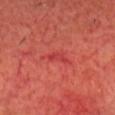On the head or neck. Imaged with cross-polarized lighting. A 15 mm close-up tile from a total-body photography series done for melanoma screening. A male patient, aged 68 to 72. The total-body-photography lesion software estimated a lesion area of about 3 mm² and a shape eccentricity near 0.8. The software also gave a nevus-likeness score of about 0/100 and a lesion-detection confidence of about 95/100.